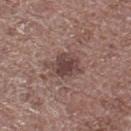• notes — catalogued during a skin exam; not biopsied
• image — ~15 mm tile from a whole-body skin photo
• site — the left lower leg
• subject — male, in their 70s
• tile lighting — white-light
• automated metrics — an average lesion color of about L≈43 a*≈18 b*≈20 (CIELAB), about 11 CIELAB-L* units darker than the surrounding skin, and a lesion-to-skin contrast of about 8.5 (normalized; higher = more distinct); a border-irregularity index near 4/10, a within-lesion color-variation index near 3.5/10, and radial color variation of about 1; a nevus-likeness score of about 35/100 and lesion-presence confidence of about 100/100
• lesion size — about 4 mm